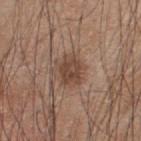Part of a total-body skin-imaging series; this lesion was reviewed on a skin check and was not flagged for biopsy.
The lesion's longest dimension is about 3.5 mm.
From the upper back.
The patient is a male aged approximately 45.
Imaged with white-light lighting.
Automated image analysis of the tile measured roughly 10 lightness units darker than nearby skin and a lesion-to-skin contrast of about 7.5 (normalized; higher = more distinct). The analysis additionally found a border-irregularity rating of about 3/10, a color-variation rating of about 3/10, and a peripheral color-asymmetry measure near 1. And it measured a classifier nevus-likeness of about 45/100 and a lesion-detection confidence of about 100/100.
A region of skin cropped from a whole-body photographic capture, roughly 15 mm wide.